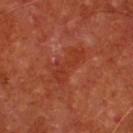Assessment: Captured during whole-body skin photography for melanoma surveillance; the lesion was not biopsied.